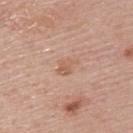  biopsy_status: not biopsied; imaged during a skin examination